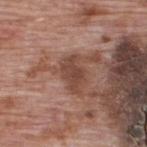The lesion was tiled from a total-body skin photograph and was not biopsied.
The lesion is located on the upper back.
A male subject aged 68 to 72.
Captured under white-light illumination.
Automated image analysis of the tile measured an eccentricity of roughly 0.8 and a shape-asymmetry score of about 0.25 (0 = symmetric). The analysis additionally found an average lesion color of about L≈45 a*≈21 b*≈26 (CIELAB), roughly 9 lightness units darker than nearby skin, and a normalized lesion–skin contrast near 7.5. The analysis additionally found a border-irregularity index near 3/10, internal color variation of about 2 on a 0–10 scale, and radial color variation of about 0.5. The analysis additionally found a classifier nevus-likeness of about 5/100 and a lesion-detection confidence of about 100/100.
A lesion tile, about 15 mm wide, cut from a 3D total-body photograph.